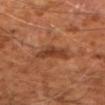Acquisition and patient details: A 15 mm crop from a total-body photograph taken for skin-cancer surveillance. The recorded lesion diameter is about 3.5 mm. The patient is a male about 60 years old. The lesion is located on the right forearm. This is a cross-polarized tile.